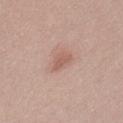Imaged during a routine full-body skin examination; the lesion was not biopsied and no histopathology is available. A lesion tile, about 15 mm wide, cut from a 3D total-body photograph. The lesion is on the leg. The patient is a female about 20 years old. Imaged with white-light lighting. Automated tile analysis of the lesion measured a lesion color around L≈58 a*≈21 b*≈25 in CIELAB, a lesion–skin lightness drop of about 8, and a lesion-to-skin contrast of about 5.5 (normalized; higher = more distinct). The software also gave a border-irregularity rating of about 4/10, a within-lesion color-variation index near 1/10, and peripheral color asymmetry of about 0.5. The analysis additionally found a nevus-likeness score of about 25/100.